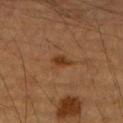The tile uses cross-polarized illumination. A 15 mm close-up tile from a total-body photography series done for melanoma screening. The patient is a male approximately 85 years of age or older. On the right upper arm.